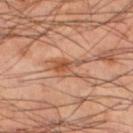Clinical impression: Captured during whole-body skin photography for melanoma surveillance; the lesion was not biopsied. Image and clinical context: Located on the left lower leg. About 4 mm across. This image is a 15 mm lesion crop taken from a total-body photograph. Automated tile analysis of the lesion measured an eccentricity of roughly 0.8 and two-axis asymmetry of about 0.45. And it measured internal color variation of about 4.5 on a 0–10 scale. Captured under cross-polarized illumination. A male patient, aged 48 to 52.